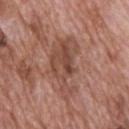Clinical impression: Imaged during a routine full-body skin examination; the lesion was not biopsied and no histopathology is available. Clinical summary: The recorded lesion diameter is about 7 mm. The total-body-photography lesion software estimated an average lesion color of about L≈48 a*≈22 b*≈27 (CIELAB) and a normalized lesion–skin contrast near 6.5. And it measured internal color variation of about 5.5 on a 0–10 scale and peripheral color asymmetry of about 2. It also reported an automated nevus-likeness rating near 0 out of 100. This is a white-light tile. The subject is a male aged 68–72. This image is a 15 mm lesion crop taken from a total-body photograph. The lesion is located on the upper back.